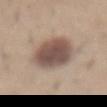Impression:
The lesion was photographed on a routine skin check and not biopsied; there is no pathology result.
Image and clinical context:
A close-up tile cropped from a whole-body skin photograph, about 15 mm across. The patient is a male aged 28–32. About 7 mm across. The tile uses white-light illumination. The lesion is located on the abdomen.